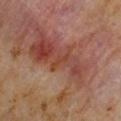Q: Is there a histopathology result?
A: total-body-photography surveillance lesion; no biopsy
Q: How was the tile lit?
A: cross-polarized illumination
Q: What kind of image is this?
A: ~15 mm tile from a whole-body skin photo
Q: Lesion size?
A: ~2.5 mm (longest diameter)
Q: Patient demographics?
A: male, aged 58 to 62
Q: What is the anatomic site?
A: the right upper arm
Q: What did automated image analysis measure?
A: a lesion–skin lightness drop of about 5; a border-irregularity rating of about 5.5/10, a color-variation rating of about 0/10, and radial color variation of about 0